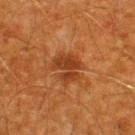An algorithmic analysis of the crop reported a footprint of about 8.5 mm², a shape eccentricity near 0.6, and two-axis asymmetry of about 0.35. And it measured roughly 9 lightness units darker than nearby skin. The analysis additionally found a classifier nevus-likeness of about 0/100 and lesion-presence confidence of about 100/100. The patient is a male aged around 60. From the upper back. This image is a 15 mm lesion crop taken from a total-body photograph. Imaged with cross-polarized lighting. Measured at roughly 3.5 mm in maximum diameter.